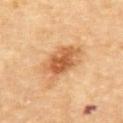Located on the upper back.
A 15 mm crop from a total-body photograph taken for skin-cancer surveillance.
The patient is a male roughly 85 years of age or older.